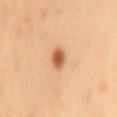Captured during whole-body skin photography for melanoma surveillance; the lesion was not biopsied.
The lesion's longest dimension is about 2.5 mm.
Cropped from a whole-body photographic skin survey; the tile spans about 15 mm.
A male patient, in their mid-50s.
The lesion is located on the mid back.
The lesion-visualizer software estimated a lesion area of about 4.5 mm² and a symmetry-axis asymmetry near 0.2. And it measured a lesion color around L≈48 a*≈20 b*≈33 in CIELAB and a lesion–skin lightness drop of about 12. The analysis additionally found an automated nevus-likeness rating near 100 out of 100 and a detector confidence of about 100 out of 100 that the crop contains a lesion.